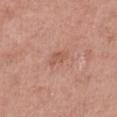Findings:
• body site: the leg
• acquisition: ~15 mm crop, total-body skin-cancer survey
• patient: female, in their 70s
• lighting: white-light
• image-analysis metrics: an automated nevus-likeness rating near 0 out of 100 and a detector confidence of about 100 out of 100 that the crop contains a lesion
• lesion size: about 2.5 mm